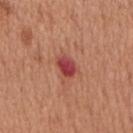| field | value |
|---|---|
| notes | catalogued during a skin exam; not biopsied |
| automated lesion analysis | an outline eccentricity of about 0.7 (0 = round, 1 = elongated) and a symmetry-axis asymmetry near 0.25; a lesion color around L≈45 a*≈35 b*≈26 in CIELAB, a lesion–skin lightness drop of about 13, and a normalized lesion–skin contrast near 10; a classifier nevus-likeness of about 0/100 and lesion-presence confidence of about 100/100 |
| illumination | white-light |
| patient | male, about 65 years old |
| body site | the mid back |
| image source | 15 mm crop, total-body photography |